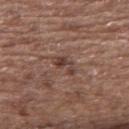This lesion was catalogued during total-body skin photography and was not selected for biopsy.
On the upper back.
A female subject about 65 years old.
A region of skin cropped from a whole-body photographic capture, roughly 15 mm wide.